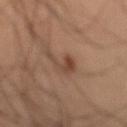{
  "biopsy_status": "not biopsied; imaged during a skin examination",
  "lesion_size": {
    "long_diameter_mm_approx": 3.0
  },
  "site": "lower back",
  "image": {
    "source": "total-body photography crop",
    "field_of_view_mm": 15
  },
  "patient": {
    "sex": "male",
    "age_approx": 65
  },
  "lighting": "cross-polarized"
}